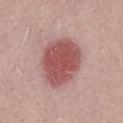Impression:
Recorded during total-body skin imaging; not selected for excision or biopsy.
Clinical summary:
The patient is a male approximately 30 years of age. Approximately 6.5 mm at its widest. Cropped from a whole-body photographic skin survey; the tile spans about 15 mm. Automated tile analysis of the lesion measured an area of roughly 23 mm² and two-axis asymmetry of about 0.1. The analysis additionally found a mean CIELAB color near L≈52 a*≈27 b*≈22, about 14 CIELAB-L* units darker than the surrounding skin, and a normalized lesion–skin contrast near 9.5. It also reported border irregularity of about 1.5 on a 0–10 scale, a color-variation rating of about 3.5/10, and a peripheral color-asymmetry measure near 1. And it measured a nevus-likeness score of about 100/100 and a detector confidence of about 100 out of 100 that the crop contains a lesion. Captured under white-light illumination.